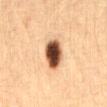Imaged during a routine full-body skin examination; the lesion was not biopsied and no histopathology is available.
The subject is a female about 30 years old.
The lesion-visualizer software estimated a mean CIELAB color near L≈47 a*≈20 b*≈31, roughly 26 lightness units darker than nearby skin, and a normalized border contrast of about 16.5.
Located on the abdomen.
Approximately 4 mm at its widest.
Imaged with cross-polarized lighting.
Cropped from a total-body skin-imaging series; the visible field is about 15 mm.